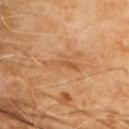{
  "biopsy_status": "not biopsied; imaged during a skin examination",
  "lesion_size": {
    "long_diameter_mm_approx": 4.5
  },
  "automated_metrics": {
    "area_mm2_approx": 4.5,
    "eccentricity": 0.95,
    "shape_asymmetry": 0.5,
    "border_irregularity_0_10": 7.0,
    "color_variation_0_10": 0.0,
    "peripheral_color_asymmetry": 0.0
  },
  "site": "chest",
  "image": {
    "source": "total-body photography crop",
    "field_of_view_mm": 15
  },
  "patient": {
    "sex": "male",
    "age_approx": 70
  },
  "lighting": "cross-polarized"
}